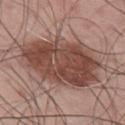follow-up: imaged on a skin check; not biopsied | lesion size: about 9 mm | subject: male, in their mid- to late 40s | image source: 15 mm crop, total-body photography | location: the upper back.